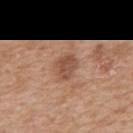Q: Is there a histopathology result?
A: catalogued during a skin exam; not biopsied
Q: What is the anatomic site?
A: the back
Q: What is the imaging modality?
A: total-body-photography crop, ~15 mm field of view
Q: What is the lesion's diameter?
A: ~3 mm (longest diameter)
Q: What lighting was used for the tile?
A: white-light
Q: Patient demographics?
A: female, aged around 55